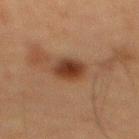Case summary:
– biopsy status · catalogued during a skin exam; not biopsied
– patient · male, about 60 years old
– tile lighting · cross-polarized
– anatomic site · the back
– image · ~15 mm crop, total-body skin-cancer survey